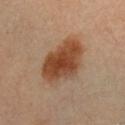tile lighting: cross-polarized | automated metrics: a lesion area of about 20 mm², an eccentricity of roughly 0.8, and two-axis asymmetry of about 0.15; border irregularity of about 2 on a 0–10 scale, internal color variation of about 5 on a 0–10 scale, and radial color variation of about 1.5; a classifier nevus-likeness of about 100/100 and a detector confidence of about 100 out of 100 that the crop contains a lesion | lesion diameter: ~6.5 mm (longest diameter) | subject: female, about 30 years old | location: the chest | acquisition: 15 mm crop, total-body photography.